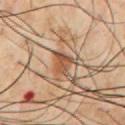notes: imaged on a skin check; not biopsied | automated lesion analysis: internal color variation of about 2.5 on a 0–10 scale and a peripheral color-asymmetry measure near 1 | lighting: cross-polarized illumination | location: the chest | subject: male, aged approximately 60 | size: ~3 mm (longest diameter) | image source: total-body-photography crop, ~15 mm field of view.